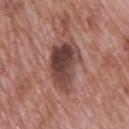Captured during whole-body skin photography for melanoma surveillance; the lesion was not biopsied. The recorded lesion diameter is about 6 mm. Automated image analysis of the tile measured an area of roughly 17 mm² and an outline eccentricity of about 0.8 (0 = round, 1 = elongated). A male patient, aged around 70. The lesion is on the upper back. A 15 mm crop from a total-body photograph taken for skin-cancer surveillance.